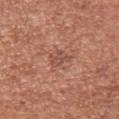| field | value |
|---|---|
| biopsy status | catalogued during a skin exam; not biopsied |
| tile lighting | white-light illumination |
| body site | the upper back |
| lesion diameter | ~2.5 mm (longest diameter) |
| imaging modality | total-body-photography crop, ~15 mm field of view |
| patient | female, about 40 years old |